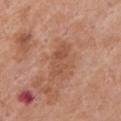notes: no biopsy performed (imaged during a skin exam) | automated lesion analysis: a footprint of about 6.5 mm² and two-axis asymmetry of about 0.4; a peripheral color-asymmetry measure near 1 | subject: female, aged around 65 | image source: ~15 mm tile from a whole-body skin photo | location: the chest.